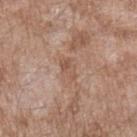Q: Was this lesion biopsied?
A: imaged on a skin check; not biopsied
Q: What is the anatomic site?
A: the left forearm
Q: What did automated image analysis measure?
A: a shape eccentricity near 0.85 and two-axis asymmetry of about 0.4; border irregularity of about 5 on a 0–10 scale, a within-lesion color-variation index near 0.5/10, and peripheral color asymmetry of about 0
Q: What lighting was used for the tile?
A: white-light illumination
Q: How large is the lesion?
A: ≈3 mm
Q: Who is the patient?
A: male, aged around 50
Q: How was this image acquired?
A: total-body-photography crop, ~15 mm field of view